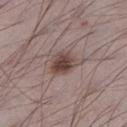Clinical impression:
Recorded during total-body skin imaging; not selected for excision or biopsy.
Background:
Measured at roughly 3.5 mm in maximum diameter. This image is a 15 mm lesion crop taken from a total-body photograph. Located on the left lower leg. Imaged with white-light lighting. An algorithmic analysis of the crop reported an area of roughly 8 mm² and an eccentricity of roughly 0.6. And it measured a mean CIELAB color near L≈44 a*≈16 b*≈20, roughly 12 lightness units darker than nearby skin, and a normalized lesion–skin contrast near 9.5. The software also gave a classifier nevus-likeness of about 90/100 and lesion-presence confidence of about 100/100. The patient is a male aged around 70.